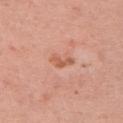biopsy status: total-body-photography surveillance lesion; no biopsy
lesion size: about 3 mm
subject: female, approximately 35 years of age
anatomic site: the left upper arm
imaging modality: total-body-photography crop, ~15 mm field of view
TBP lesion metrics: a footprint of about 3.5 mm² and an outline eccentricity of about 0.85 (0 = round, 1 = elongated); about 9 CIELAB-L* units darker than the surrounding skin and a normalized lesion–skin contrast near 6.5; a within-lesion color-variation index near 0/10
lighting: white-light